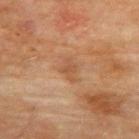Case summary:
* image — 15 mm crop, total-body photography
* patient — female, in their 80s
* diameter — ≈3 mm
* anatomic site — the upper back
* lighting — cross-polarized